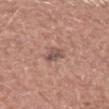Assessment:
The lesion was tiled from a total-body skin photograph and was not biopsied.
Clinical summary:
A male subject aged approximately 65. This image is a 15 mm lesion crop taken from a total-body photograph. About 2.5 mm across. From the left upper arm.